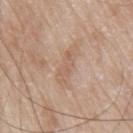Imaged during a routine full-body skin examination; the lesion was not biopsied and no histopathology is available.
A close-up tile cropped from a whole-body skin photograph, about 15 mm across.
Imaged with white-light lighting.
Automated image analysis of the tile measured a border-irregularity rating of about 3.5/10 and a peripheral color-asymmetry measure near 1.
The patient is a male approximately 80 years of age.
The recorded lesion diameter is about 5 mm.
The lesion is on the front of the torso.